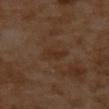The lesion was tiled from a total-body skin photograph and was not biopsied.
A lesion tile, about 15 mm wide, cut from a 3D total-body photograph.
Imaged with cross-polarized lighting.
The lesion is on the upper back.
A female patient, approximately 55 years of age.
The recorded lesion diameter is about 3 mm.